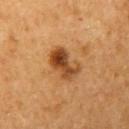Findings:
* biopsy status · catalogued during a skin exam; not biopsied
* image source · 15 mm crop, total-body photography
* anatomic site · the left upper arm
* illumination · cross-polarized illumination
* patient · female, aged around 50
* size · about 4 mm